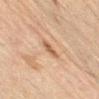workup: total-body-photography surveillance lesion; no biopsy | acquisition: 15 mm crop, total-body photography | size: about 3 mm | site: the front of the torso | patient: male, aged 68–72 | illumination: cross-polarized illumination.